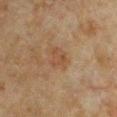Impression: The lesion was tiled from a total-body skin photograph and was not biopsied.